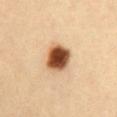- location · the abdomen
- image source · ~15 mm tile from a whole-body skin photo
- tile lighting · cross-polarized illumination
- TBP lesion metrics · a classifier nevus-likeness of about 100/100 and lesion-presence confidence of about 100/100
- lesion diameter · about 3.5 mm
- patient · female, aged around 35